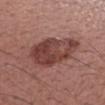Notes:
- workup — imaged on a skin check; not biopsied
- patient — female, roughly 55 years of age
- imaging modality — ~15 mm tile from a whole-body skin photo
- anatomic site — the right forearm